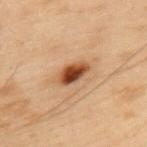{"biopsy_status": "not biopsied; imaged during a skin examination", "lesion_size": {"long_diameter_mm_approx": 3.5}, "image": {"source": "total-body photography crop", "field_of_view_mm": 15}, "site": "mid back", "lighting": "cross-polarized", "patient": {"sex": "male", "age_approx": 55}}